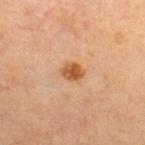{
  "image": {
    "source": "total-body photography crop",
    "field_of_view_mm": 15
  },
  "site": "upper back",
  "patient": {
    "sex": "male",
    "age_approx": 60
  }
}